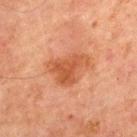Recorded during total-body skin imaging; not selected for excision or biopsy.